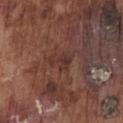Q: Patient demographics?
A: male, aged approximately 75
Q: What kind of image is this?
A: ~15 mm crop, total-body skin-cancer survey
Q: Where on the body is the lesion?
A: the chest